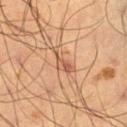<record>
  <image>
    <source>total-body photography crop</source>
    <field_of_view_mm>15</field_of_view_mm>
  </image>
  <patient>
    <sex>male</sex>
    <age_approx>65</age_approx>
  </patient>
  <site>right thigh</site>
</record>